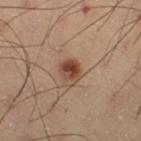Q: Is there a histopathology result?
A: catalogued during a skin exam; not biopsied